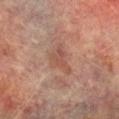The lesion was photographed on a routine skin check and not biopsied; there is no pathology result. The lesion's longest dimension is about 4 mm. The subject is a male about 75 years old. This image is a 15 mm lesion crop taken from a total-body photograph. The tile uses cross-polarized illumination. From the right lower leg.